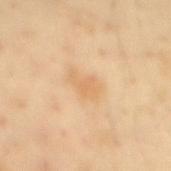Recorded during total-body skin imaging; not selected for excision or biopsy. The total-body-photography lesion software estimated an automated nevus-likeness rating near 0 out of 100 and a detector confidence of about 100 out of 100 that the crop contains a lesion. The patient is a male aged around 40. About 3 mm across. A 15 mm crop from a total-body photograph taken for skin-cancer surveillance. From the back. Imaged with cross-polarized lighting.